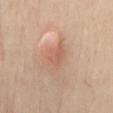Impression:
The lesion was photographed on a routine skin check and not biopsied; there is no pathology result.
Image and clinical context:
A female subject, aged approximately 55. This is a cross-polarized tile. Automated tile analysis of the lesion measured an area of roughly 4.5 mm², an eccentricity of roughly 0.75, and two-axis asymmetry of about 0.4. It also reported an average lesion color of about L≈60 a*≈22 b*≈31 (CIELAB), a lesion–skin lightness drop of about 7, and a normalized border contrast of about 5. The software also gave a color-variation rating of about 2.5/10 and a peripheral color-asymmetry measure near 1. The analysis additionally found an automated nevus-likeness rating near 15 out of 100. Measured at roughly 3 mm in maximum diameter. Located on the mid back. This image is a 15 mm lesion crop taken from a total-body photograph.